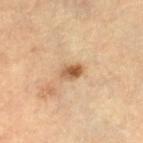subject = female, aged 63 to 67 | site = the leg | image-analysis metrics = border irregularity of about 2 on a 0–10 scale, internal color variation of about 6.5 on a 0–10 scale, and radial color variation of about 2.5; a classifier nevus-likeness of about 90/100 | acquisition = total-body-photography crop, ~15 mm field of view | lesion size = ~2.5 mm (longest diameter).